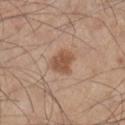* biopsy status · catalogued during a skin exam; not biopsied
* image · ~15 mm tile from a whole-body skin photo
* TBP lesion metrics · a lesion area of about 6 mm², a shape eccentricity near 0.7, and two-axis asymmetry of about 0.25; a border-irregularity index near 2.5/10, internal color variation of about 2.5 on a 0–10 scale, and radial color variation of about 1
* subject · male, in their mid- to late 40s
* site · the leg
* illumination · white-light illumination